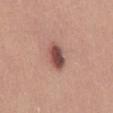Clinical impression:
No biopsy was performed on this lesion — it was imaged during a full skin examination and was not determined to be concerning.
Image and clinical context:
The recorded lesion diameter is about 3.5 mm. A close-up tile cropped from a whole-body skin photograph, about 15 mm across. Located on the back. This is a white-light tile. A male patient, about 25 years old. Automated image analysis of the tile measured an area of roughly 7 mm². The software also gave a mean CIELAB color near L≈49 a*≈23 b*≈23, a lesion–skin lightness drop of about 15, and a lesion-to-skin contrast of about 10.5 (normalized; higher = more distinct). The analysis additionally found a nevus-likeness score of about 95/100 and a detector confidence of about 100 out of 100 that the crop contains a lesion.